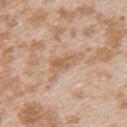Assessment:
The lesion was tiled from a total-body skin photograph and was not biopsied.
Background:
A region of skin cropped from a whole-body photographic capture, roughly 15 mm wide. The total-body-photography lesion software estimated a lesion–skin lightness drop of about 8 and a normalized lesion–skin contrast near 6. Captured under white-light illumination. The lesion is located on the left upper arm. A female patient in their mid-20s. About 5 mm across.